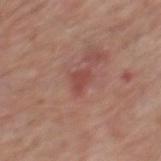notes — no biopsy performed (imaged during a skin exam) | site — the back | image — ~15 mm crop, total-body skin-cancer survey | subject — male, in their 80s.